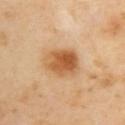{
  "biopsy_status": "not biopsied; imaged during a skin examination",
  "lesion_size": {
    "long_diameter_mm_approx": 4.5
  },
  "image": {
    "source": "total-body photography crop",
    "field_of_view_mm": 15
  },
  "patient": {
    "sex": "female",
    "age_approx": 40
  },
  "site": "upper back",
  "lighting": "cross-polarized",
  "automated_metrics": {
    "eccentricity": 0.65,
    "cielab_L": 59,
    "cielab_a": 23,
    "cielab_b": 41,
    "vs_skin_darker_L": 13.0,
    "vs_skin_contrast_norm": 9.0
  }
}